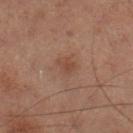follow-up: catalogued during a skin exam; not biopsied | illumination: cross-polarized | body site: the right lower leg | automated lesion analysis: a lesion area of about 4 mm², an eccentricity of roughly 0.65, and two-axis asymmetry of about 0.25 | diameter: ~2.5 mm (longest diameter) | patient: male, aged approximately 60 | image: 15 mm crop, total-body photography.